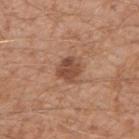The lesion was photographed on a routine skin check and not biopsied; there is no pathology result. A lesion tile, about 15 mm wide, cut from a 3D total-body photograph. The lesion is on the right upper arm. The subject is a male aged 28–32. Captured under white-light illumination.